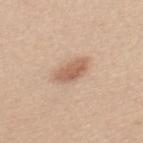This lesion was catalogued during total-body skin photography and was not selected for biopsy. Measured at roughly 4 mm in maximum diameter. A region of skin cropped from a whole-body photographic capture, roughly 15 mm wide. The lesion is on the back. A female patient roughly 40 years of age.